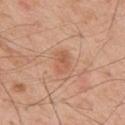Part of a total-body skin-imaging series; this lesion was reviewed on a skin check and was not flagged for biopsy.
The recorded lesion diameter is about 3 mm.
A male subject aged approximately 55.
Cropped from a total-body skin-imaging series; the visible field is about 15 mm.
Located on the mid back.
Imaged with white-light lighting.